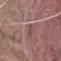Findings:
* biopsy status · imaged on a skin check; not biopsied
* subject · male, aged around 80
* imaging modality · 15 mm crop, total-body photography
* lighting · white-light
* lesion diameter · ~3 mm (longest diameter)